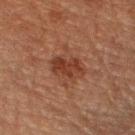follow-up — total-body-photography surveillance lesion; no biopsy | site — the right forearm | acquisition — total-body-photography crop, ~15 mm field of view | patient — male, approximately 75 years of age.